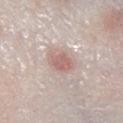Findings:
- biopsy status: catalogued during a skin exam; not biopsied
- image: total-body-photography crop, ~15 mm field of view
- anatomic site: the leg
- lighting: white-light illumination
- lesion size: ≈3.5 mm
- subject: male, approximately 65 years of age
- image-analysis metrics: border irregularity of about 1.5 on a 0–10 scale and a peripheral color-asymmetry measure near 1